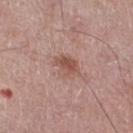Impression:
This lesion was catalogued during total-body skin photography and was not selected for biopsy.
Acquisition and patient details:
A region of skin cropped from a whole-body photographic capture, roughly 15 mm wide. A male patient about 75 years old. This is a white-light tile. Located on the right thigh.